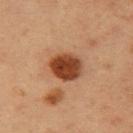image source: ~15 mm crop, total-body skin-cancer survey | subject: male, about 35 years old | site: the right upper arm.